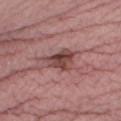Part of a total-body skin-imaging series; this lesion was reviewed on a skin check and was not flagged for biopsy.